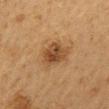Q: Was this lesion biopsied?
A: imaged on a skin check; not biopsied
Q: Where on the body is the lesion?
A: the back
Q: Illumination type?
A: cross-polarized illumination
Q: Automated lesion metrics?
A: an average lesion color of about L≈38 a*≈17 b*≈31 (CIELAB), a lesion–skin lightness drop of about 10, and a lesion-to-skin contrast of about 8.5 (normalized; higher = more distinct)
Q: What are the patient's age and sex?
A: male, aged 58–62
Q: What kind of image is this?
A: 15 mm crop, total-body photography